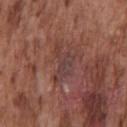The lesion was tiled from a total-body skin photograph and was not biopsied. Imaged with white-light lighting. The patient is a male aged around 75. A roughly 15 mm field-of-view crop from a total-body skin photograph. The lesion is located on the chest. Measured at roughly 4.5 mm in maximum diameter.